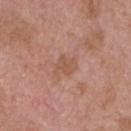Q: Was this lesion biopsied?
A: no biopsy performed (imaged during a skin exam)
Q: What are the patient's age and sex?
A: male, aged 73–77
Q: What is the imaging modality?
A: ~15 mm crop, total-body skin-cancer survey
Q: What lighting was used for the tile?
A: white-light
Q: What is the lesion's diameter?
A: ≈3 mm
Q: Lesion location?
A: the head or neck